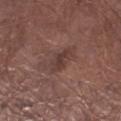Q: Is there a histopathology result?
A: total-body-photography surveillance lesion; no biopsy
Q: What is the lesion's diameter?
A: about 3.5 mm
Q: Patient demographics?
A: male, aged around 55
Q: What kind of image is this?
A: ~15 mm tile from a whole-body skin photo
Q: Automated lesion metrics?
A: an average lesion color of about L≈38 a*≈19 b*≈21 (CIELAB) and about 7 CIELAB-L* units darker than the surrounding skin
Q: Lesion location?
A: the leg